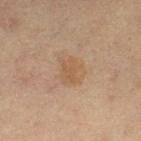biopsy status: no biopsy performed (imaged during a skin exam)
patient: female, about 20 years old
automated lesion analysis: an area of roughly 8.5 mm² and two-axis asymmetry of about 0.2; border irregularity of about 2 on a 0–10 scale and internal color variation of about 2 on a 0–10 scale; a nevus-likeness score of about 5/100 and a detector confidence of about 100 out of 100 that the crop contains a lesion
tile lighting: cross-polarized illumination
image: ~15 mm tile from a whole-body skin photo
anatomic site: the left thigh